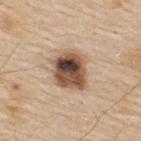Assessment: The lesion was photographed on a routine skin check and not biopsied; there is no pathology result. Image and clinical context: The tile uses white-light illumination. An algorithmic analysis of the crop reported a footprint of about 15 mm², a shape eccentricity near 0.55, and a symmetry-axis asymmetry near 0.2. The analysis additionally found radial color variation of about 4.5. A 15 mm crop from a total-body photograph taken for skin-cancer surveillance. The patient is a male approximately 80 years of age. Approximately 4.5 mm at its widest. Located on the upper back.